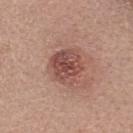* patient — female, about 35 years old
* location — the head or neck
* imaging modality — ~15 mm crop, total-body skin-cancer survey